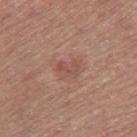Q: Was a biopsy performed?
A: total-body-photography surveillance lesion; no biopsy
Q: What is the anatomic site?
A: the right thigh
Q: What are the patient's age and sex?
A: female, aged 63 to 67
Q: What is the lesion's diameter?
A: about 3 mm
Q: What kind of image is this?
A: ~15 mm crop, total-body skin-cancer survey